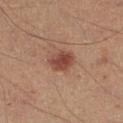No biopsy was performed on this lesion — it was imaged during a full skin examination and was not determined to be concerning. The lesion is on the right lower leg. A male patient, aged 48 to 52. The lesion's longest dimension is about 3 mm. Cropped from a whole-body photographic skin survey; the tile spans about 15 mm.